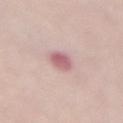notes — imaged on a skin check; not biopsied | illumination — white-light | patient — female, in their mid- to late 60s | TBP lesion metrics — an average lesion color of about L≈60 a*≈26 b*≈18 (CIELAB) and a lesion–skin lightness drop of about 12; border irregularity of about 1.5 on a 0–10 scale, internal color variation of about 3 on a 0–10 scale, and peripheral color asymmetry of about 1 | acquisition — 15 mm crop, total-body photography | lesion size — ≈3 mm | anatomic site — the mid back.